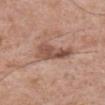The tile uses white-light illumination.
A male patient aged 68–72.
Measured at roughly 5 mm in maximum diameter.
Cropped from a whole-body photographic skin survey; the tile spans about 15 mm.
On the abdomen.